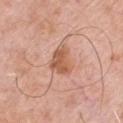Imaged during a routine full-body skin examination; the lesion was not biopsied and no histopathology is available. The lesion is on the chest. A male patient aged approximately 80. A close-up tile cropped from a whole-body skin photograph, about 15 mm across. Longest diameter approximately 4 mm. Captured under white-light illumination.